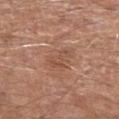Impression: Captured during whole-body skin photography for melanoma surveillance; the lesion was not biopsied. Acquisition and patient details: The patient is a male approximately 65 years of age. A close-up tile cropped from a whole-body skin photograph, about 15 mm across. From the left forearm.